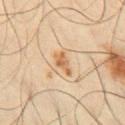| field | value |
|---|---|
| follow-up | no biopsy performed (imaged during a skin exam) |
| TBP lesion metrics | a lesion area of about 4 mm² and two-axis asymmetry of about 0.4 |
| image source | ~15 mm crop, total-body skin-cancer survey |
| subject | male, aged around 65 |
| lighting | cross-polarized illumination |
| site | the chest |
| size | ≈3 mm |